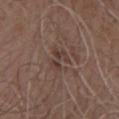Part of a total-body skin-imaging series; this lesion was reviewed on a skin check and was not flagged for biopsy. A male subject approximately 70 years of age. From the chest. Longest diameter approximately 2.5 mm. Captured under white-light illumination. A 15 mm close-up extracted from a 3D total-body photography capture.